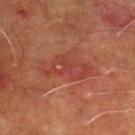{
  "image": {
    "source": "total-body photography crop",
    "field_of_view_mm": 15
  },
  "lesion_size": {
    "long_diameter_mm_approx": 5.5
  },
  "patient": {
    "sex": "male",
    "age_approx": 70
  },
  "site": "left lower leg"
}